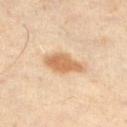The lesion was photographed on a routine skin check and not biopsied; there is no pathology result. A lesion tile, about 15 mm wide, cut from a 3D total-body photograph. A male patient, roughly 60 years of age. From the right thigh. About 4.5 mm across. Captured under cross-polarized illumination.